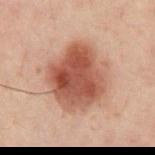* biopsy status: catalogued during a skin exam; not biopsied
* lesion size: about 6.5 mm
* subject: male, aged 38–42
* imaging modality: total-body-photography crop, ~15 mm field of view
* location: the chest